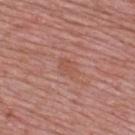{
  "biopsy_status": "not biopsied; imaged during a skin examination",
  "site": "upper back",
  "image": {
    "source": "total-body photography crop",
    "field_of_view_mm": 15
  },
  "patient": {
    "sex": "male",
    "age_approx": 50
  }
}